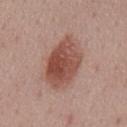No biopsy was performed on this lesion — it was imaged during a full skin examination and was not determined to be concerning. The recorded lesion diameter is about 7 mm. The tile uses white-light illumination. Cropped from a total-body skin-imaging series; the visible field is about 15 mm. A male patient, aged approximately 55. An algorithmic analysis of the crop reported a border-irregularity rating of about 2/10 and peripheral color asymmetry of about 2. And it measured an automated nevus-likeness rating near 100 out of 100 and a detector confidence of about 100 out of 100 that the crop contains a lesion. The lesion is located on the back.